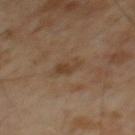workup: imaged on a skin check; not biopsied
imaging modality: ~15 mm crop, total-body skin-cancer survey
patient: male, in their mid-60s